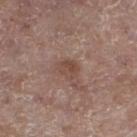diameter: ~2.5 mm (longest diameter) | tile lighting: white-light | TBP lesion metrics: a footprint of about 4.5 mm², a shape eccentricity near 0.6, and a symmetry-axis asymmetry near 0.25; roughly 7 lightness units darker than nearby skin and a normalized border contrast of about 6; a border-irregularity rating of about 2.5/10 and a color-variation rating of about 2.5/10; a nevus-likeness score of about 0/100 and lesion-presence confidence of about 100/100 | patient: female, in their mid- to late 80s or older | site: the left lower leg | imaging modality: 15 mm crop, total-body photography.